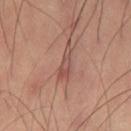Recorded during total-body skin imaging; not selected for excision or biopsy.